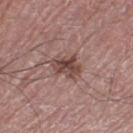Q: Was this lesion biopsied?
A: total-body-photography surveillance lesion; no biopsy
Q: What kind of image is this?
A: ~15 mm tile from a whole-body skin photo
Q: Patient demographics?
A: male, aged 73–77
Q: Where on the body is the lesion?
A: the left thigh
Q: Lesion size?
A: ~3.5 mm (longest diameter)